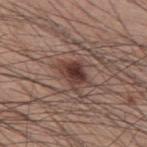Clinical impression:
The lesion was photographed on a routine skin check and not biopsied; there is no pathology result.
Acquisition and patient details:
The total-body-photography lesion software estimated a lesion area of about 6.5 mm² and an eccentricity of roughly 0.7. The analysis additionally found an average lesion color of about L≈37 a*≈20 b*≈22 (CIELAB), a lesion–skin lightness drop of about 12, and a normalized lesion–skin contrast near 10.5. And it measured a border-irregularity rating of about 3.5/10, a within-lesion color-variation index near 5/10, and peripheral color asymmetry of about 1.5. The software also gave a lesion-detection confidence of about 100/100. About 3.5 mm across. The lesion is on the mid back. The patient is a male aged approximately 60. This is a white-light tile. A 15 mm crop from a total-body photograph taken for skin-cancer surveillance.